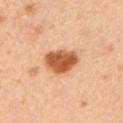Imaged during a routine full-body skin examination; the lesion was not biopsied and no histopathology is available. The tile uses cross-polarized illumination. The recorded lesion diameter is about 4 mm. The lesion is on the arm. A 15 mm close-up tile from a total-body photography series done for melanoma screening. The subject is a male in their mid- to late 50s. An algorithmic analysis of the crop reported roughly 18 lightness units darker than nearby skin and a lesion-to-skin contrast of about 11.5 (normalized; higher = more distinct).